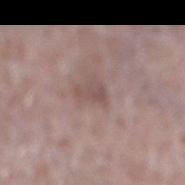{
  "biopsy_status": "not biopsied; imaged during a skin examination"
}